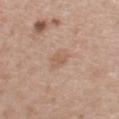Impression: The lesion was tiled from a total-body skin photograph and was not biopsied. Background: Longest diameter approximately 2.5 mm. On the chest. The lesion-visualizer software estimated an area of roughly 4 mm², a shape eccentricity near 0.7, and a symmetry-axis asymmetry near 0.2. The software also gave a classifier nevus-likeness of about 0/100 and a lesion-detection confidence of about 100/100. A female subject, in their 40s. The tile uses white-light illumination. A 15 mm close-up tile from a total-body photography series done for melanoma screening.